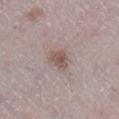<lesion>
<site>right lower leg</site>
<patient>
  <sex>female</sex>
  <age_approx>50</age_approx>
</patient>
<image>
  <source>total-body photography crop</source>
  <field_of_view_mm>15</field_of_view_mm>
</image>
<automated_metrics>
  <area_mm2_approx>4.5</area_mm2_approx>
  <eccentricity>0.75</eccentricity>
  <shape_asymmetry>0.3</shape_asymmetry>
  <border_irregularity_0_10>3.0</border_irregularity_0_10>
  <color_variation_0_10>2.5</color_variation_0_10>
  <peripheral_color_asymmetry>1.0</peripheral_color_asymmetry>
  <nevus_likeness_0_100>75</nevus_likeness_0_100>
  <lesion_detection_confidence_0_100>100</lesion_detection_confidence_0_100>
</automated_metrics>
</lesion>